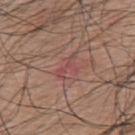Q: Was this lesion biopsied?
A: total-body-photography surveillance lesion; no biopsy
Q: Lesion location?
A: the mid back
Q: What is the lesion's diameter?
A: about 3.5 mm
Q: Automated lesion metrics?
A: a border-irregularity rating of about 7.5/10, internal color variation of about 0 on a 0–10 scale, and radial color variation of about 0; a classifier nevus-likeness of about 0/100 and a lesion-detection confidence of about 90/100
Q: Patient demographics?
A: male, approximately 75 years of age
Q: What kind of image is this?
A: ~15 mm tile from a whole-body skin photo
Q: What lighting was used for the tile?
A: white-light illumination